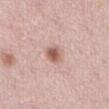Part of a total-body skin-imaging series; this lesion was reviewed on a skin check and was not flagged for biopsy.
Longest diameter approximately 2.5 mm.
Automated image analysis of the tile measured roughly 12 lightness units darker than nearby skin and a lesion-to-skin contrast of about 8 (normalized; higher = more distinct). The analysis additionally found a border-irregularity rating of about 2/10 and a peripheral color-asymmetry measure near 1. The software also gave a nevus-likeness score of about 85/100 and a detector confidence of about 100 out of 100 that the crop contains a lesion.
The subject is a female approximately 65 years of age.
A 15 mm close-up tile from a total-body photography series done for melanoma screening.
The tile uses white-light illumination.
From the abdomen.